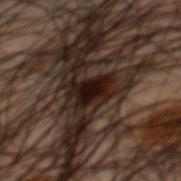Imaged during a routine full-body skin examination; the lesion was not biopsied and no histopathology is available.
The patient is a male about 50 years old.
Measured at roughly 3.5 mm in maximum diameter.
A 15 mm close-up tile from a total-body photography series done for melanoma screening.
Captured under cross-polarized illumination.
From the mid back.
Automated tile analysis of the lesion measured a lesion–skin lightness drop of about 10 and a normalized border contrast of about 15.5. It also reported border irregularity of about 4.5 on a 0–10 scale, a color-variation rating of about 3/10, and peripheral color asymmetry of about 1. It also reported a classifier nevus-likeness of about 100/100 and lesion-presence confidence of about 50/100.